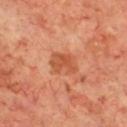Imaged with cross-polarized lighting.
Located on the chest.
A 15 mm crop from a total-body photograph taken for skin-cancer surveillance.
An algorithmic analysis of the crop reported a lesion area of about 6.5 mm², an outline eccentricity of about 0.7 (0 = round, 1 = elongated), and two-axis asymmetry of about 0.3. The software also gave an average lesion color of about L≈52 a*≈29 b*≈37 (CIELAB) and roughly 9 lightness units darker than nearby skin. The software also gave a nevus-likeness score of about 0/100.
The recorded lesion diameter is about 3.5 mm.
The subject is a male aged 68 to 72.